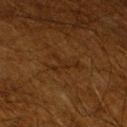| field | value |
|---|---|
| biopsy status | imaged on a skin check; not biopsied |
| patient | male, aged around 60 |
| automated lesion analysis | a footprint of about 5.5 mm², a shape eccentricity near 0.85, and a shape-asymmetry score of about 0.75 (0 = symmetric); an automated nevus-likeness rating near 0 out of 100 and a lesion-detection confidence of about 80/100 |
| anatomic site | the left lower leg |
| tile lighting | cross-polarized |
| imaging modality | ~15 mm tile from a whole-body skin photo |
| lesion size | ~4 mm (longest diameter) |